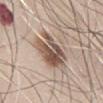Q: Was a biopsy performed?
A: imaged on a skin check; not biopsied
Q: What are the patient's age and sex?
A: male, aged approximately 65
Q: Illumination type?
A: white-light
Q: What is the anatomic site?
A: the abdomen
Q: What kind of image is this?
A: ~15 mm tile from a whole-body skin photo